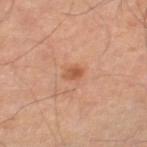Measured at roughly 2 mm in maximum diameter. Captured under cross-polarized illumination. An algorithmic analysis of the crop reported a lesion area of about 2.5 mm² and two-axis asymmetry of about 0.2. The analysis additionally found a lesion color around L≈51 a*≈24 b*≈34 in CIELAB and roughly 9 lightness units darker than nearby skin. The analysis additionally found a nevus-likeness score of about 75/100 and lesion-presence confidence of about 100/100. A male subject approximately 65 years of age. On the right thigh. A lesion tile, about 15 mm wide, cut from a 3D total-body photograph.